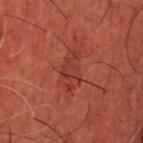Clinical impression:
No biopsy was performed on this lesion — it was imaged during a full skin examination and was not determined to be concerning.
Clinical summary:
Located on the head or neck. Captured under cross-polarized illumination. A male patient, aged 58–62. A region of skin cropped from a whole-body photographic capture, roughly 15 mm wide. Measured at roughly 4 mm in maximum diameter.